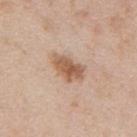Findings:
- workup: imaged on a skin check; not biopsied
- illumination: white-light illumination
- image source: 15 mm crop, total-body photography
- lesion diameter: about 4.5 mm
- subject: male, aged 58–62
- location: the back